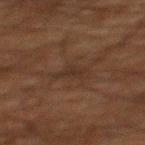Notes:
– acquisition · ~15 mm crop, total-body skin-cancer survey
– site · the mid back
– illumination · cross-polarized
– patient · male, roughly 60 years of age